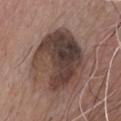<lesion>
<biopsy_status>not biopsied; imaged during a skin examination</biopsy_status>
<image>
  <source>total-body photography crop</source>
  <field_of_view_mm>15</field_of_view_mm>
</image>
<patient>
  <sex>male</sex>
  <age_approx>75</age_approx>
</patient>
<site>chest</site>
<lighting>white-light</lighting>
<lesion_size>
  <long_diameter_mm_approx>8.0</long_diameter_mm_approx>
</lesion_size>
<automated_metrics>
  <eccentricity>0.4</eccentricity>
  <cielab_L>40</cielab_L>
  <cielab_a>15</cielab_a>
  <cielab_b>21</cielab_b>
  <vs_skin_darker_L>13.0</vs_skin_darker_L>
  <vs_skin_contrast_norm>10.5</vs_skin_contrast_norm>
  <border_irregularity_0_10>2.0</border_irregularity_0_10>
  <peripheral_color_asymmetry>3.5</peripheral_color_asymmetry>
  <nevus_likeness_0_100>0</nevus_likeness_0_100>
  <lesion_detection_confidence_0_100>100</lesion_detection_confidence_0_100>
</automated_metrics>
</lesion>